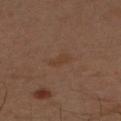Background: Approximately 2.5 mm at its widest. The lesion-visualizer software estimated an outline eccentricity of about 0.85 (0 = round, 1 = elongated) and two-axis asymmetry of about 0.3. And it measured a border-irregularity rating of about 3/10 and a color-variation rating of about 1/10. The software also gave an automated nevus-likeness rating near 0 out of 100. A region of skin cropped from a whole-body photographic capture, roughly 15 mm wide. A male subject aged 43–47. Located on the right upper arm.